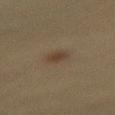The lesion was tiled from a total-body skin photograph and was not biopsied.
The lesion is located on the left thigh.
The tile uses cross-polarized illumination.
A female patient, in their 50s.
A region of skin cropped from a whole-body photographic capture, roughly 15 mm wide.
About 3.5 mm across.